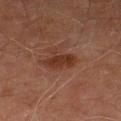<record>
  <biopsy_status>not biopsied; imaged during a skin examination</biopsy_status>
  <automated_metrics>
    <area_mm2_approx>6.5</area_mm2_approx>
    <eccentricity>0.8</eccentricity>
    <cielab_L>27</cielab_L>
    <cielab_a>19</cielab_a>
    <cielab_b>24</cielab_b>
    <vs_skin_contrast_norm>8.0</vs_skin_contrast_norm>
    <border_irregularity_0_10>4.5</border_irregularity_0_10>
    <color_variation_0_10>2.0</color_variation_0_10>
    <peripheral_color_asymmetry>0.5</peripheral_color_asymmetry>
  </automated_metrics>
  <image>
    <source>total-body photography crop</source>
    <field_of_view_mm>15</field_of_view_mm>
  </image>
  <patient>
    <sex>male</sex>
    <age_approx>70</age_approx>
  </patient>
  <site>right lower leg</site>
  <lighting>cross-polarized</lighting>
  <lesion_size>
    <long_diameter_mm_approx>3.5</long_diameter_mm_approx>
  </lesion_size>
</record>